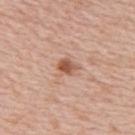Clinical impression:
The lesion was photographed on a routine skin check and not biopsied; there is no pathology result.
Clinical summary:
An algorithmic analysis of the crop reported a lesion area of about 3.5 mm², an outline eccentricity of about 0.7 (0 = round, 1 = elongated), and two-axis asymmetry of about 0.25. The analysis additionally found roughly 12 lightness units darker than nearby skin and a normalized lesion–skin contrast near 8.5. It also reported lesion-presence confidence of about 100/100. A 15 mm crop from a total-body photograph taken for skin-cancer surveillance. The lesion's longest dimension is about 2.5 mm. The subject is a male about 50 years old. On the upper back.